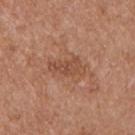workup: imaged on a skin check; not biopsied | acquisition: total-body-photography crop, ~15 mm field of view | subject: male, aged 53–57 | tile lighting: white-light illumination | lesion size: about 4 mm | location: the left upper arm.